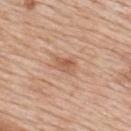Clinical impression: Recorded during total-body skin imaging; not selected for excision or biopsy. Clinical summary: The tile uses white-light illumination. A region of skin cropped from a whole-body photographic capture, roughly 15 mm wide. Measured at roughly 3 mm in maximum diameter. A male patient, aged around 60. Automated image analysis of the tile measured a mean CIELAB color near L≈58 a*≈22 b*≈32 and a normalized lesion–skin contrast near 6. It also reported border irregularity of about 2.5 on a 0–10 scale and internal color variation of about 3.5 on a 0–10 scale. The lesion is on the upper back.